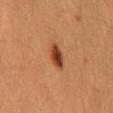biopsy status — total-body-photography surveillance lesion; no biopsy | lighting — cross-polarized | automated lesion analysis — an area of roughly 5 mm² and an eccentricity of roughly 0.8; a lesion color around L≈34 a*≈22 b*≈29 in CIELAB and a normalized border contrast of about 10.5 | patient — male, aged 48–52 | lesion size — ~3 mm (longest diameter) | location — the mid back | acquisition — ~15 mm tile from a whole-body skin photo.